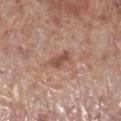{
  "lighting": "white-light",
  "image": {
    "source": "total-body photography crop",
    "field_of_view_mm": 15
  },
  "site": "leg",
  "patient": {
    "sex": "male",
    "age_approx": 70
  }
}